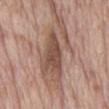Findings:
* follow-up — catalogued during a skin exam; not biopsied
* illumination — white-light
* body site — the abdomen
* acquisition — total-body-photography crop, ~15 mm field of view
* patient — male, roughly 70 years of age
* TBP lesion metrics — an average lesion color of about L≈52 a*≈18 b*≈26 (CIELAB), a lesion–skin lightness drop of about 11, and a lesion-to-skin contrast of about 8 (normalized; higher = more distinct); a border-irregularity index near 8/10, internal color variation of about 4.5 on a 0–10 scale, and a peripheral color-asymmetry measure near 1.5; a classifier nevus-likeness of about 0/100 and lesion-presence confidence of about 50/100